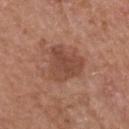A 15 mm close-up tile from a total-body photography series done for melanoma screening.
On the head or neck.
Automated tile analysis of the lesion measured a border-irregularity index near 3.5/10, a within-lesion color-variation index near 3/10, and peripheral color asymmetry of about 1. And it measured an automated nevus-likeness rating near 15 out of 100 and a lesion-detection confidence of about 100/100.
The lesion's longest dimension is about 5.5 mm.
Imaged with white-light lighting.
A male patient, aged 53–57.